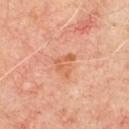Q: Was a biopsy performed?
A: imaged on a skin check; not biopsied
Q: Who is the patient?
A: male, aged 63 to 67
Q: How was this image acquired?
A: 15 mm crop, total-body photography
Q: What is the anatomic site?
A: the chest